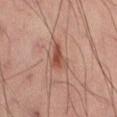Impression: Imaged during a routine full-body skin examination; the lesion was not biopsied and no histopathology is available. Acquisition and patient details: A male subject aged around 50. The lesion's longest dimension is about 3.5 mm. The lesion is located on the left thigh. Cropped from a whole-body photographic skin survey; the tile spans about 15 mm. Automated tile analysis of the lesion measured an average lesion color of about L≈45 a*≈21 b*≈25 (CIELAB) and roughly 10 lightness units darker than nearby skin. It also reported border irregularity of about 4.5 on a 0–10 scale, a within-lesion color-variation index near 4/10, and radial color variation of about 1. It also reported a nevus-likeness score of about 60/100 and lesion-presence confidence of about 100/100.